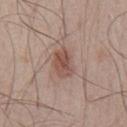Impression: Recorded during total-body skin imaging; not selected for excision or biopsy. Clinical summary: The lesion is located on the chest. Measured at roughly 3.5 mm in maximum diameter. This is a white-light tile. A male patient aged around 50. A lesion tile, about 15 mm wide, cut from a 3D total-body photograph.